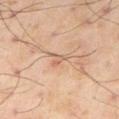{"biopsy_status": "not biopsied; imaged during a skin examination", "automated_metrics": {"area_mm2_approx": 2.5, "eccentricity": 0.9, "shape_asymmetry": 0.4, "cielab_L": 64, "cielab_a": 20, "cielab_b": 31, "vs_skin_contrast_norm": 5.0, "border_irregularity_0_10": 4.0, "peripheral_color_asymmetry": 0.0, "nevus_likeness_0_100": 0, "lesion_detection_confidence_0_100": 80}, "lesion_size": {"long_diameter_mm_approx": 2.5}, "site": "right thigh", "lighting": "cross-polarized", "image": {"source": "total-body photography crop", "field_of_view_mm": 15}, "patient": {"sex": "male", "age_approx": 55}}